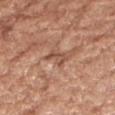Q: Was this lesion biopsied?
A: imaged on a skin check; not biopsied
Q: What is the imaging modality?
A: ~15 mm tile from a whole-body skin photo
Q: Lesion size?
A: ~3 mm (longest diameter)
Q: Illumination type?
A: white-light illumination
Q: Where on the body is the lesion?
A: the right forearm
Q: What are the patient's age and sex?
A: female, aged 73–77
Q: Automated lesion metrics?
A: an area of roughly 4 mm², an outline eccentricity of about 0.85 (0 = round, 1 = elongated), and a symmetry-axis asymmetry near 0.45